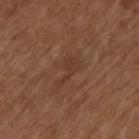biopsy status: no biopsy performed (imaged during a skin exam) | patient: male, aged 73 to 77 | TBP lesion metrics: a lesion area of about 4.5 mm², an outline eccentricity of about 0.85 (0 = round, 1 = elongated), and two-axis asymmetry of about 0.65; border irregularity of about 7 on a 0–10 scale, internal color variation of about 1.5 on a 0–10 scale, and radial color variation of about 0.5; a classifier nevus-likeness of about 0/100 | size: ~3.5 mm (longest diameter) | illumination: white-light | location: the arm | image: 15 mm crop, total-body photography.